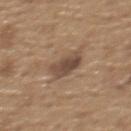Captured under white-light illumination.
A male subject, aged approximately 65.
Measured at roughly 4 mm in maximum diameter.
A 15 mm close-up tile from a total-body photography series done for melanoma screening.
The lesion-visualizer software estimated a mean CIELAB color near L≈46 a*≈16 b*≈26.
On the upper back.